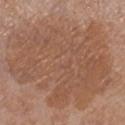Notes:
– biopsy status: imaged on a skin check; not biopsied
– automated lesion analysis: an average lesion color of about L≈52 a*≈20 b*≈29 (CIELAB), about 8 CIELAB-L* units darker than the surrounding skin, and a normalized lesion–skin contrast near 6; a border-irregularity rating of about 5.5/10, a color-variation rating of about 4/10, and peripheral color asymmetry of about 1.5; an automated nevus-likeness rating near 5 out of 100 and lesion-presence confidence of about 100/100
– anatomic site: the right lower leg
– lighting: white-light
– patient: female, about 55 years old
– image: total-body-photography crop, ~15 mm field of view